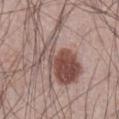{"biopsy_status": "not biopsied; imaged during a skin examination", "lighting": "white-light", "site": "abdomen", "automated_metrics": {"cielab_L": 50, "cielab_a": 18, "cielab_b": 21, "vs_skin_darker_L": 12.0, "vs_skin_contrast_norm": 8.5, "color_variation_0_10": 7.0, "nevus_likeness_0_100": 100}, "lesion_size": {"long_diameter_mm_approx": 9.0}, "image": {"source": "total-body photography crop", "field_of_view_mm": 15}, "patient": {"sex": "male", "age_approx": 65}}